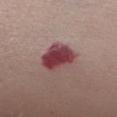workup=no biopsy performed (imaged during a skin exam) | image=total-body-photography crop, ~15 mm field of view | lesion size=~4.5 mm (longest diameter) | site=the abdomen | patient=female, aged 48 to 52 | lighting=white-light illumination.